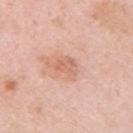Part of a total-body skin-imaging series; this lesion was reviewed on a skin check and was not flagged for biopsy. Cropped from a whole-body photographic skin survey; the tile spans about 15 mm. Automated tile analysis of the lesion measured a lesion area of about 5 mm², a shape eccentricity near 0.7, and a symmetry-axis asymmetry near 0.35. The software also gave border irregularity of about 3.5 on a 0–10 scale, a within-lesion color-variation index near 2/10, and a peripheral color-asymmetry measure near 0.5. And it measured a nevus-likeness score of about 0/100. The patient is a female in their mid-60s. On the right upper arm.